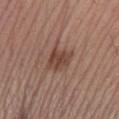Imaged during a routine full-body skin examination; the lesion was not biopsied and no histopathology is available. Captured under white-light illumination. Cropped from a total-body skin-imaging series; the visible field is about 15 mm. The subject is a female aged 58–62. The lesion's longest dimension is about 3.5 mm. The total-body-photography lesion software estimated an area of roughly 7 mm², an eccentricity of roughly 0.75, and two-axis asymmetry of about 0.35. The analysis additionally found a lesion color around L≈43 a*≈20 b*≈25 in CIELAB and about 10 CIELAB-L* units darker than the surrounding skin. The analysis additionally found a border-irregularity index near 3.5/10 and a within-lesion color-variation index near 2.5/10. It also reported a classifier nevus-likeness of about 75/100 and lesion-presence confidence of about 100/100. From the left forearm.